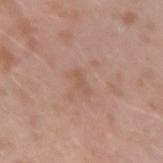Clinical impression: No biopsy was performed on this lesion — it was imaged during a full skin examination and was not determined to be concerning. Context: A lesion tile, about 15 mm wide, cut from a 3D total-body photograph. The subject is a male aged 38 to 42. On the left forearm.